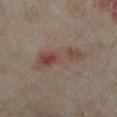Q: Is there a histopathology result?
A: total-body-photography surveillance lesion; no biopsy
Q: What did automated image analysis measure?
A: an area of roughly 11 mm² and an outline eccentricity of about 0.95 (0 = round, 1 = elongated); a within-lesion color-variation index near 8/10 and radial color variation of about 2; a nevus-likeness score of about 15/100 and a detector confidence of about 100 out of 100 that the crop contains a lesion
Q: What is the imaging modality?
A: ~15 mm crop, total-body skin-cancer survey
Q: Who is the patient?
A: female, aged 33–37
Q: What is the lesion's diameter?
A: ~6.5 mm (longest diameter)
Q: How was the tile lit?
A: cross-polarized
Q: Lesion location?
A: the leg